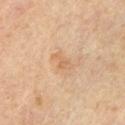Captured during whole-body skin photography for melanoma surveillance; the lesion was not biopsied.
Automated image analysis of the tile measured about 7 CIELAB-L* units darker than the surrounding skin and a lesion-to-skin contrast of about 5 (normalized; higher = more distinct).
The lesion is located on the left upper arm.
A region of skin cropped from a whole-body photographic capture, roughly 15 mm wide.
The lesion's longest dimension is about 2.5 mm.
Captured under cross-polarized illumination.
The patient is a male aged 53–57.